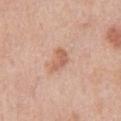Impression:
No biopsy was performed on this lesion — it was imaged during a full skin examination and was not determined to be concerning.
Image and clinical context:
The tile uses white-light illumination. A close-up tile cropped from a whole-body skin photograph, about 15 mm across. The total-body-photography lesion software estimated a footprint of about 4 mm², an outline eccentricity of about 0.8 (0 = round, 1 = elongated), and a symmetry-axis asymmetry near 0.45. And it measured an average lesion color of about L≈61 a*≈23 b*≈31 (CIELAB) and a normalized lesion–skin contrast near 6.5. It also reported border irregularity of about 4.5 on a 0–10 scale, a within-lesion color-variation index near 1.5/10, and radial color variation of about 0.5. The lesion is located on the abdomen. Approximately 3 mm at its widest. The patient is a male aged around 70.